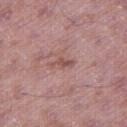Q: Was a biopsy performed?
A: imaged on a skin check; not biopsied
Q: Lesion size?
A: about 3 mm
Q: Who is the patient?
A: male, approximately 50 years of age
Q: Lesion location?
A: the right thigh
Q: What kind of image is this?
A: ~15 mm tile from a whole-body skin photo